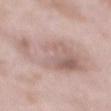Captured during whole-body skin photography for melanoma surveillance; the lesion was not biopsied. The recorded lesion diameter is about 9 mm. Automated tile analysis of the lesion measured a lesion color around L≈63 a*≈17 b*≈22 in CIELAB. The analysis additionally found a lesion-detection confidence of about 100/100. A 15 mm close-up extracted from a 3D total-body photography capture. The subject is a male roughly 55 years of age. From the mid back. Imaged with white-light lighting.